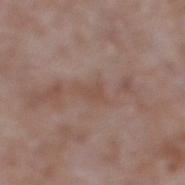The lesion was tiled from a total-body skin photograph and was not biopsied. Captured under white-light illumination. The lesion is located on the right lower leg. The recorded lesion diameter is about 3 mm. The total-body-photography lesion software estimated an outline eccentricity of about 0.9 (0 = round, 1 = elongated) and a symmetry-axis asymmetry near 0.5. The software also gave a mean CIELAB color near L≈49 a*≈18 b*≈25, roughly 6 lightness units darker than nearby skin, and a normalized border contrast of about 5.5. The software also gave a nevus-likeness score of about 0/100. The subject is a male about 60 years old. Cropped from a whole-body photographic skin survey; the tile spans about 15 mm.